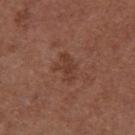Part of a total-body skin-imaging series; this lesion was reviewed on a skin check and was not flagged for biopsy.
A male subject aged 53–57.
On the arm.
Automated image analysis of the tile measured a border-irregularity rating of about 5/10, internal color variation of about 1 on a 0–10 scale, and radial color variation of about 0.5.
The recorded lesion diameter is about 3 mm.
A 15 mm close-up tile from a total-body photography series done for melanoma screening.